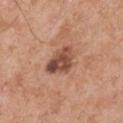{"biopsy_status": "not biopsied; imaged during a skin examination", "lighting": "white-light", "lesion_size": {"long_diameter_mm_approx": 4.5}, "image": {"source": "total-body photography crop", "field_of_view_mm": 15}, "patient": {"sex": "male", "age_approx": 55}, "site": "right upper arm", "automated_metrics": {"area_mm2_approx": 9.0, "eccentricity": 0.7, "shape_asymmetry": 0.3, "border_irregularity_0_10": 3.0, "color_variation_0_10": 8.0, "nevus_likeness_0_100": 10, "lesion_detection_confidence_0_100": 100}}